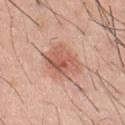follow-up = imaged on a skin check; not biopsied
diameter = about 5 mm
tile lighting = white-light
patient = male, aged 43–47
acquisition = 15 mm crop, total-body photography
location = the chest